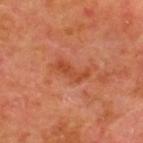{"biopsy_status": "not biopsied; imaged during a skin examination", "image": {"source": "total-body photography crop", "field_of_view_mm": 15}, "site": "chest", "patient": {"sex": "male", "age_approx": 45}}